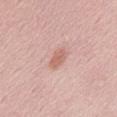follow-up = imaged on a skin check; not biopsied | image = total-body-photography crop, ~15 mm field of view | lesion size = ≈3 mm | subject = male, roughly 60 years of age | site = the abdomen.